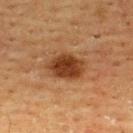  patient:
    sex: male
    age_approx: 60
  lighting: cross-polarized
  image:
    source: total-body photography crop
    field_of_view_mm: 15
  lesion_size:
    long_diameter_mm_approx: 5.0
  site: back